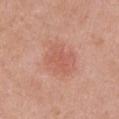No biopsy was performed on this lesion — it was imaged during a full skin examination and was not determined to be concerning. A 15 mm close-up extracted from a 3D total-body photography capture. From the left upper arm. Captured under white-light illumination. Longest diameter approximately 5.5 mm. The patient is a female roughly 50 years of age.